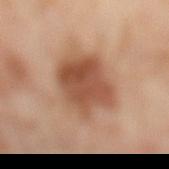Imaged during a routine full-body skin examination; the lesion was not biopsied and no histopathology is available.
Imaged with cross-polarized lighting.
From the left lower leg.
A close-up tile cropped from a whole-body skin photograph, about 15 mm across.
A female subject in their 50s.
Approximately 6 mm at its widest.